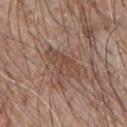Assessment:
Captured during whole-body skin photography for melanoma surveillance; the lesion was not biopsied.
Acquisition and patient details:
A lesion tile, about 15 mm wide, cut from a 3D total-body photograph. Approximately 5 mm at its widest. A male subject aged approximately 65. The lesion is located on the chest.